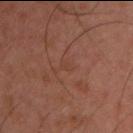  biopsy_status: not biopsied; imaged during a skin examination
  image:
    source: total-body photography crop
    field_of_view_mm: 15
  patient:
    sex: male
    age_approx: 45
  site: right upper arm
  automated_metrics:
    cielab_L: 38
    cielab_a: 21
    cielab_b: 26
    vs_skin_darker_L: 3.0
    vs_skin_contrast_norm: 3.5
    lesion_detection_confidence_0_100: 95